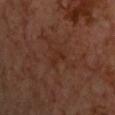The lesion was photographed on a routine skin check and not biopsied; there is no pathology result.
A 15 mm crop from a total-body photograph taken for skin-cancer surveillance.
The lesion is on the chest.
Imaged with cross-polarized lighting.
A male patient, about 70 years old.
Measured at roughly 2.5 mm in maximum diameter.